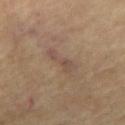Impression: Captured during whole-body skin photography for melanoma surveillance; the lesion was not biopsied. Image and clinical context: This is a cross-polarized tile. Automated tile analysis of the lesion measured an area of roughly 4.5 mm², an eccentricity of roughly 0.95, and two-axis asymmetry of about 0.4. And it measured about 7 CIELAB-L* units darker than the surrounding skin and a normalized lesion–skin contrast near 6. The software also gave a border-irregularity rating of about 5.5/10, internal color variation of about 1.5 on a 0–10 scale, and radial color variation of about 0.5. The analysis additionally found an automated nevus-likeness rating near 0 out of 100 and a detector confidence of about 95 out of 100 that the crop contains a lesion. From the mid back. A close-up tile cropped from a whole-body skin photograph, about 15 mm across. Approximately 4 mm at its widest. A male subject, aged around 70.